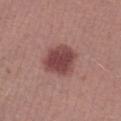This lesion was catalogued during total-body skin photography and was not selected for biopsy. The patient is a male aged 28 to 32. The recorded lesion diameter is about 4 mm. A 15 mm crop from a total-body photograph taken for skin-cancer surveillance. Imaged with white-light lighting.